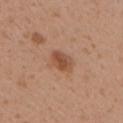Q: Illumination type?
A: white-light illumination
Q: What is the imaging modality?
A: ~15 mm crop, total-body skin-cancer survey
Q: Where on the body is the lesion?
A: the right upper arm
Q: Patient demographics?
A: female, in their 30s
Q: Lesion size?
A: ~3 mm (longest diameter)